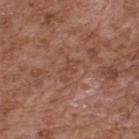Assessment:
This lesion was catalogued during total-body skin photography and was not selected for biopsy.
Acquisition and patient details:
A male subject about 75 years old. The lesion is located on the upper back. The recorded lesion diameter is about 3 mm. A lesion tile, about 15 mm wide, cut from a 3D total-body photograph. The total-body-photography lesion software estimated a lesion–skin lightness drop of about 5. The tile uses white-light illumination.